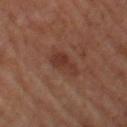Impression:
This lesion was catalogued during total-body skin photography and was not selected for biopsy.
Acquisition and patient details:
A female patient, aged 63 to 67. Automated tile analysis of the lesion measured an automated nevus-likeness rating near 5 out of 100. A lesion tile, about 15 mm wide, cut from a 3D total-body photograph. Captured under cross-polarized illumination. On the leg.